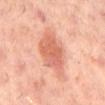  biopsy_status: not biopsied; imaged during a skin examination
  site: mid back
  lighting: cross-polarized
  lesion_size:
    long_diameter_mm_approx: 6.5
  image:
    source: total-body photography crop
    field_of_view_mm: 15
  patient:
    sex: male
    age_approx: 60